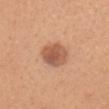Clinical impression: Imaged during a routine full-body skin examination; the lesion was not biopsied and no histopathology is available. Image and clinical context: The subject is a female in their mid- to late 20s. Cropped from a whole-body photographic skin survey; the tile spans about 15 mm. Captured under white-light illumination. Located on the head or neck. About 4 mm across. Automated image analysis of the tile measured a mean CIELAB color near L≈56 a*≈24 b*≈33, roughly 12 lightness units darker than nearby skin, and a normalized lesion–skin contrast near 8. And it measured a border-irregularity rating of about 1.5/10, a color-variation rating of about 3.5/10, and radial color variation of about 1.